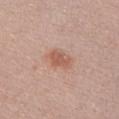The lesion was photographed on a routine skin check and not biopsied; there is no pathology result.
A female subject in their mid-20s.
This is a white-light tile.
The lesion is located on the chest.
Cropped from a total-body skin-imaging series; the visible field is about 15 mm.